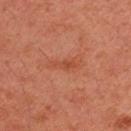biopsy status — catalogued during a skin exam; not biopsied
image-analysis metrics — a mean CIELAB color near L≈45 a*≈28 b*≈34, a lesion–skin lightness drop of about 6, and a normalized border contrast of about 5.5
location — the right upper arm
imaging modality — ~15 mm tile from a whole-body skin photo
subject — male, roughly 45 years of age
lighting — cross-polarized illumination
lesion size — ≈3 mm